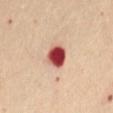| key | value |
|---|---|
| follow-up | catalogued during a skin exam; not biopsied |
| illumination | cross-polarized illumination |
| site | the front of the torso |
| automated lesion analysis | an area of roughly 7.5 mm² and a symmetry-axis asymmetry near 0.2 |
| subject | female, in their 60s |
| image source | ~15 mm tile from a whole-body skin photo |